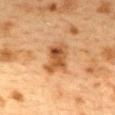{
  "biopsy_status": "not biopsied; imaged during a skin examination",
  "image": {
    "source": "total-body photography crop",
    "field_of_view_mm": 15
  },
  "patient": {
    "sex": "female",
    "age_approx": 40
  },
  "automated_metrics": {
    "area_mm2_approx": 10.0,
    "eccentricity": 0.7,
    "shape_asymmetry": 0.3,
    "cielab_L": 46,
    "cielab_a": 21,
    "cielab_b": 35,
    "vs_skin_contrast_norm": 8.5,
    "border_irregularity_0_10": 3.0,
    "color_variation_0_10": 5.5,
    "peripheral_color_asymmetry": 1.5,
    "nevus_likeness_0_100": 85,
    "lesion_detection_confidence_0_100": 100
  },
  "lesion_size": {
    "long_diameter_mm_approx": 4.5
  },
  "site": "mid back",
  "lighting": "cross-polarized"
}